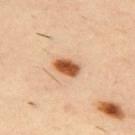notes — imaged on a skin check; not biopsied | patient — male, in their 40s | size — about 3 mm | image — ~15 mm crop, total-body skin-cancer survey | location — the upper back.